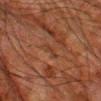biopsy status: catalogued during a skin exam; not biopsied | lesion size: about 2.5 mm | anatomic site: the leg | tile lighting: cross-polarized illumination | subject: male, about 80 years old | acquisition: 15 mm crop, total-body photography.